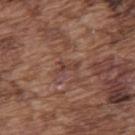A male patient, in their mid-70s.
About 3 mm across.
A 15 mm crop from a total-body photograph taken for skin-cancer surveillance.
The lesion is located on the upper back.
The tile uses white-light illumination.